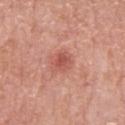Assessment: The lesion was tiled from a total-body skin photograph and was not biopsied. Clinical summary: This is a white-light tile. The lesion is on the mid back. The subject is a male roughly 65 years of age. A roughly 15 mm field-of-view crop from a total-body skin photograph. Measured at roughly 2.5 mm in maximum diameter.